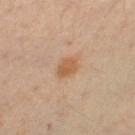follow-up: total-body-photography surveillance lesion; no biopsy | site: the right leg | illumination: cross-polarized | subject: female, in their 40s | imaging modality: total-body-photography crop, ~15 mm field of view.